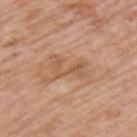Acquisition and patient details: From the back. A male subject, in their mid-70s. Captured under white-light illumination. A lesion tile, about 15 mm wide, cut from a 3D total-body photograph. Measured at roughly 4 mm in maximum diameter.